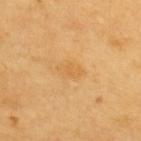Recorded during total-body skin imaging; not selected for excision or biopsy. This is a cross-polarized tile. Located on the upper back. Cropped from a total-body skin-imaging series; the visible field is about 15 mm. The subject is a male approximately 60 years of age. The lesion's longest dimension is about 3 mm.